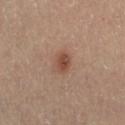{"biopsy_status": "not biopsied; imaged during a skin examination", "patient": {"sex": "female", "age_approx": 45}, "image": {"source": "total-body photography crop", "field_of_view_mm": 15}, "site": "right thigh"}